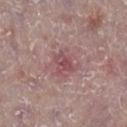subject — male, approximately 75 years of age | tile lighting — white-light illumination | image source — ~15 mm crop, total-body skin-cancer survey | size — about 2.5 mm | anatomic site — the right lower leg.